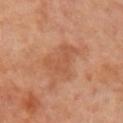Q: Was this lesion biopsied?
A: no biopsy performed (imaged during a skin exam)
Q: What did automated image analysis measure?
A: an area of roughly 9 mm² and a shape-asymmetry score of about 0.55 (0 = symmetric); an average lesion color of about L≈52 a*≈24 b*≈34 (CIELAB), roughly 7 lightness units darker than nearby skin, and a normalized border contrast of about 5; a nevus-likeness score of about 0/100 and lesion-presence confidence of about 100/100
Q: How was the tile lit?
A: cross-polarized
Q: How was this image acquired?
A: 15 mm crop, total-body photography
Q: What is the lesion's diameter?
A: ~4.5 mm (longest diameter)
Q: Lesion location?
A: the left arm
Q: Who is the patient?
A: female, in their mid- to late 60s